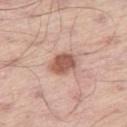Recorded during total-body skin imaging; not selected for excision or biopsy. The lesion is on the right thigh. This image is a 15 mm lesion crop taken from a total-body photograph. The subject is a male aged around 55.